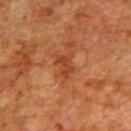This lesion was catalogued during total-body skin photography and was not selected for biopsy. A 15 mm close-up tile from a total-body photography series done for melanoma screening. From the upper back. A male subject roughly 80 years of age.